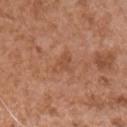workup: total-body-photography surveillance lesion; no biopsy | subject: male, roughly 75 years of age | image-analysis metrics: a normalized border contrast of about 5; a border-irregularity rating of about 4.5/10, internal color variation of about 0.5 on a 0–10 scale, and peripheral color asymmetry of about 0 | location: the front of the torso | lighting: white-light illumination | imaging modality: ~15 mm tile from a whole-body skin photo | size: about 3 mm.